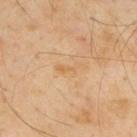This lesion was catalogued during total-body skin photography and was not selected for biopsy. Approximately 3 mm at its widest. A 15 mm close-up extracted from a 3D total-body photography capture. Imaged with cross-polarized lighting. Located on the upper back. The total-body-photography lesion software estimated an area of roughly 3.5 mm², a shape eccentricity near 0.9, and a shape-asymmetry score of about 0.6 (0 = symmetric). It also reported a lesion–skin lightness drop of about 5. And it measured border irregularity of about 6.5 on a 0–10 scale, a color-variation rating of about 0.5/10, and radial color variation of about 0. And it measured a classifier nevus-likeness of about 0/100. A male patient about 55 years old.